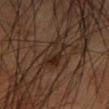| feature | finding |
|---|---|
| follow-up | no biopsy performed (imaged during a skin exam) |
| image source | ~15 mm tile from a whole-body skin photo |
| lesion size | about 4 mm |
| automated lesion analysis | a footprint of about 8 mm² and two-axis asymmetry of about 0.45; border irregularity of about 5 on a 0–10 scale, a within-lesion color-variation index near 7/10, and peripheral color asymmetry of about 2.5 |
| location | the left forearm |
| subject | male, about 60 years old |